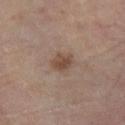Part of a total-body skin-imaging series; this lesion was reviewed on a skin check and was not flagged for biopsy.
The recorded lesion diameter is about 2.5 mm.
An algorithmic analysis of the crop reported a lesion area of about 4.5 mm², an outline eccentricity of about 0.65 (0 = round, 1 = elongated), and two-axis asymmetry of about 0.25. And it measured a border-irregularity index near 2.5/10 and a color-variation rating of about 2/10.
A 15 mm close-up extracted from a 3D total-body photography capture.
A male patient, aged 63–67.